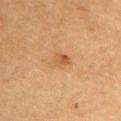Case summary:
- diameter — about 3 mm
- anatomic site — the upper back
- acquisition — ~15 mm crop, total-body skin-cancer survey
- illumination — cross-polarized
- patient — female, aged 58 to 62